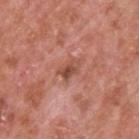Q: Was this lesion biopsied?
A: catalogued during a skin exam; not biopsied
Q: What lighting was used for the tile?
A: white-light
Q: Automated lesion metrics?
A: an average lesion color of about L≈49 a*≈26 b*≈29 (CIELAB) and a lesion–skin lightness drop of about 11; an automated nevus-likeness rating near 10 out of 100
Q: How was this image acquired?
A: ~15 mm crop, total-body skin-cancer survey
Q: Who is the patient?
A: male, aged 63 to 67
Q: How large is the lesion?
A: about 2.5 mm
Q: Lesion location?
A: the upper back